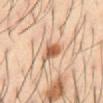Background: Cropped from a whole-body photographic skin survey; the tile spans about 15 mm. The tile uses cross-polarized illumination. A male patient approximately 40 years of age. Approximately 2.5 mm at its widest. On the abdomen.